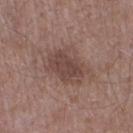<lesion>
<biopsy_status>not biopsied; imaged during a skin examination</biopsy_status>
<patient>
  <sex>male</sex>
  <age_approx>45</age_approx>
</patient>
<automated_metrics>
  <area_mm2_approx>12.0</area_mm2_approx>
  <eccentricity>0.75</eccentricity>
  <shape_asymmetry>0.2</shape_asymmetry>
  <lesion_detection_confidence_0_100>100</lesion_detection_confidence_0_100>
</automated_metrics>
<lighting>white-light</lighting>
<image>
  <source>total-body photography crop</source>
  <field_of_view_mm>15</field_of_view_mm>
</image>
<site>right lower leg</site>
<lesion_size>
  <long_diameter_mm_approx>5.0</long_diameter_mm_approx>
</lesion_size>
</lesion>